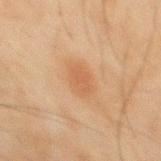biopsy status: no biopsy performed (imaged during a skin exam) | subject: male, in their mid-40s | image-analysis metrics: a classifier nevus-likeness of about 60/100 and lesion-presence confidence of about 100/100 | image source: ~15 mm tile from a whole-body skin photo | location: the mid back | diameter: ~3.5 mm (longest diameter) | illumination: cross-polarized.